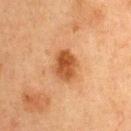patient=female, aged 58 to 62
location=the upper back
acquisition=15 mm crop, total-body photography
lighting=cross-polarized illumination